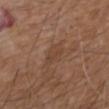{
  "biopsy_status": "not biopsied; imaged during a skin examination",
  "patient": {
    "sex": "male",
    "age_approx": 75
  },
  "site": "upper back",
  "image": {
    "source": "total-body photography crop",
    "field_of_view_mm": 15
  }
}